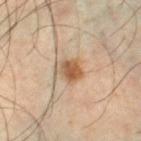Q: Was a biopsy performed?
A: catalogued during a skin exam; not biopsied
Q: Who is the patient?
A: male, aged approximately 50
Q: How was this image acquired?
A: total-body-photography crop, ~15 mm field of view
Q: Lesion size?
A: ≈3 mm
Q: How was the tile lit?
A: cross-polarized
Q: What is the anatomic site?
A: the right lower leg